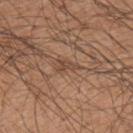Q: Where on the body is the lesion?
A: the left upper arm
Q: What are the patient's age and sex?
A: male, aged 58 to 62
Q: What did automated image analysis measure?
A: an area of roughly 3 mm², a shape eccentricity near 0.9, and a symmetry-axis asymmetry near 0.5; an average lesion color of about L≈46 a*≈18 b*≈29 (CIELAB) and a lesion–skin lightness drop of about 8; a border-irregularity index near 6/10, a color-variation rating of about 0/10, and a peripheral color-asymmetry measure near 0
Q: What kind of image is this?
A: total-body-photography crop, ~15 mm field of view
Q: How was the tile lit?
A: white-light illumination
Q: What is the lesion's diameter?
A: ≈3 mm